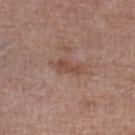notes — catalogued during a skin exam; not biopsied | body site — the right lower leg | imaging modality — total-body-photography crop, ~15 mm field of view | patient — female, approximately 70 years of age.